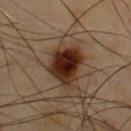Background:
A 15 mm close-up tile from a total-body photography series done for melanoma screening. An algorithmic analysis of the crop reported a footprint of about 13 mm² and a shape eccentricity near 0.7. The software also gave a mean CIELAB color near L≈20 a*≈16 b*≈21 and a normalized border contrast of about 16. Captured under cross-polarized illumination. Approximately 5 mm at its widest. A male patient, about 55 years old. Located on the chest.